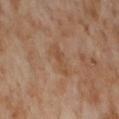This lesion was catalogued during total-body skin photography and was not selected for biopsy. On the right thigh. A female patient, approximately 55 years of age. The recorded lesion diameter is about 4 mm. Imaged with cross-polarized lighting. A region of skin cropped from a whole-body photographic capture, roughly 15 mm wide.